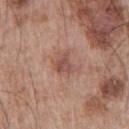Q: Was a biopsy performed?
A: imaged on a skin check; not biopsied
Q: What are the patient's age and sex?
A: male, about 65 years old
Q: What kind of image is this?
A: total-body-photography crop, ~15 mm field of view
Q: Lesion location?
A: the left upper arm